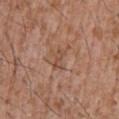No biopsy was performed on this lesion — it was imaged during a full skin examination and was not determined to be concerning.
Approximately 2.5 mm at its widest.
Located on the front of the torso.
Automated tile analysis of the lesion measured a lesion-detection confidence of about 100/100.
A lesion tile, about 15 mm wide, cut from a 3D total-body photograph.
This is a white-light tile.
A male subject, aged 43 to 47.